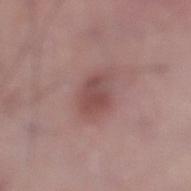follow-up: no biopsy performed (imaged during a skin exam)
image: ~15 mm tile from a whole-body skin photo
automated lesion analysis: a footprint of about 6.5 mm², an outline eccentricity of about 0.6 (0 = round, 1 = elongated), and a symmetry-axis asymmetry near 0.2; a border-irregularity index near 2/10 and a peripheral color-asymmetry measure near 1
body site: the left lower leg
patient: male, aged approximately 50
size: ~3 mm (longest diameter)